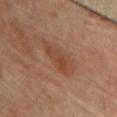notes: catalogued during a skin exam; not biopsied
automated lesion analysis: a shape eccentricity near 0.95 and a shape-asymmetry score of about 0.3 (0 = symmetric)
anatomic site: the chest
subject: female, approximately 65 years of age
tile lighting: cross-polarized
image: total-body-photography crop, ~15 mm field of view
lesion diameter: ~5.5 mm (longest diameter)